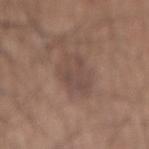Clinical impression:
Part of a total-body skin-imaging series; this lesion was reviewed on a skin check and was not flagged for biopsy.
Background:
Captured under white-light illumination. The subject is a male in their mid-70s. A close-up tile cropped from a whole-body skin photograph, about 15 mm across. The lesion is located on the back.